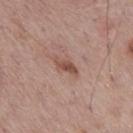No biopsy was performed on this lesion — it was imaged during a full skin examination and was not determined to be concerning.
A 15 mm close-up tile from a total-body photography series done for melanoma screening.
From the back.
The recorded lesion diameter is about 3.5 mm.
A male subject approximately 70 years of age.
The tile uses white-light illumination.
Automated tile analysis of the lesion measured an area of roughly 3.5 mm² and an eccentricity of roughly 0.9. The analysis additionally found a lesion–skin lightness drop of about 11. The software also gave a border-irregularity index near 3.5/10.